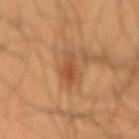| field | value |
|---|---|
| biopsy status | no biopsy performed (imaged during a skin exam) |
| lesion size | about 4 mm |
| automated metrics | a lesion area of about 6 mm², a shape eccentricity near 0.9, and a shape-asymmetry score of about 0.35 (0 = symmetric); a mean CIELAB color near L≈49 a*≈23 b*≈37 and a normalized lesion–skin contrast near 6.5; a border-irregularity rating of about 4/10, a color-variation rating of about 2/10, and peripheral color asymmetry of about 0.5; an automated nevus-likeness rating near 80 out of 100 |
| image source | total-body-photography crop, ~15 mm field of view |
| tile lighting | cross-polarized illumination |
| anatomic site | the mid back |
| subject | male, about 65 years old |